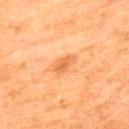| feature | finding |
|---|---|
| workup | total-body-photography surveillance lesion; no biopsy |
| site | the upper back |
| lesion size | ≈3 mm |
| patient | male, aged 78–82 |
| imaging modality | 15 mm crop, total-body photography |